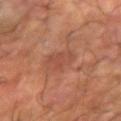| key | value |
|---|---|
| workup | imaged on a skin check; not biopsied |
| subject | male, roughly 60 years of age |
| image source | ~15 mm crop, total-body skin-cancer survey |
| lighting | cross-polarized |
| anatomic site | the right forearm |
| size | ≈3 mm |